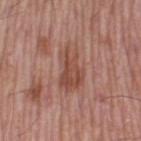<tbp_lesion>
<site>leg</site>
<patient>
  <sex>male</sex>
  <age_approx>55</age_approx>
</patient>
<lighting>white-light</lighting>
<image>
  <source>total-body photography crop</source>
  <field_of_view_mm>15</field_of_view_mm>
</image>
</tbp_lesion>